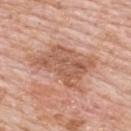{
  "lighting": "white-light",
  "patient": {
    "sex": "male",
    "age_approx": 80
  },
  "lesion_size": {
    "long_diameter_mm_approx": 7.0
  },
  "site": "upper back",
  "image": {
    "source": "total-body photography crop",
    "field_of_view_mm": 15
  },
  "automated_metrics": {
    "area_mm2_approx": 21.0,
    "eccentricity": 0.75,
    "border_irregularity_0_10": 5.0,
    "color_variation_0_10": 4.0,
    "peripheral_color_asymmetry": 1.5
  }
}